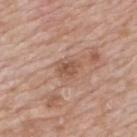Impression:
The lesion was photographed on a routine skin check and not biopsied; there is no pathology result.
Clinical summary:
Cropped from a total-body skin-imaging series; the visible field is about 15 mm. The tile uses white-light illumination. A male patient, aged 58 to 62. The lesion's longest dimension is about 2.5 mm. The lesion is located on the mid back.